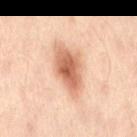Captured during whole-body skin photography for melanoma surveillance; the lesion was not biopsied. The lesion's longest dimension is about 6 mm. A 15 mm crop from a total-body photograph taken for skin-cancer surveillance. The subject is a female about 55 years old. The lesion is located on the lower back. The tile uses cross-polarized illumination.